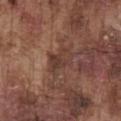The lesion was photographed on a routine skin check and not biopsied; there is no pathology result. Imaged with white-light lighting. A male patient, approximately 75 years of age. Automated image analysis of the tile measured a mean CIELAB color near L≈38 a*≈19 b*≈24 and a lesion–skin lightness drop of about 8. The analysis additionally found a border-irregularity rating of about 3.5/10, internal color variation of about 4.5 on a 0–10 scale, and peripheral color asymmetry of about 2. It also reported a nevus-likeness score of about 0/100 and lesion-presence confidence of about 60/100. This image is a 15 mm lesion crop taken from a total-body photograph. The lesion is on the chest. Longest diameter approximately 3.5 mm.